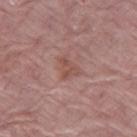Q: Was a biopsy performed?
A: imaged on a skin check; not biopsied
Q: Lesion location?
A: the left thigh
Q: Illumination type?
A: white-light
Q: Who is the patient?
A: female, approximately 65 years of age
Q: How was this image acquired?
A: total-body-photography crop, ~15 mm field of view
Q: How large is the lesion?
A: ≈3 mm